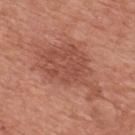The lesion was photographed on a routine skin check and not biopsied; there is no pathology result.
A 15 mm crop from a total-body photograph taken for skin-cancer surveillance.
The lesion-visualizer software estimated a shape eccentricity near 0.8 and two-axis asymmetry of about 0.4. It also reported an average lesion color of about L≈50 a*≈26 b*≈29 (CIELAB). The software also gave a border-irregularity index near 6/10, a color-variation rating of about 3/10, and a peripheral color-asymmetry measure near 1. The software also gave a classifier nevus-likeness of about 35/100 and lesion-presence confidence of about 100/100.
The recorded lesion diameter is about 8 mm.
This is a white-light tile.
A male patient, aged approximately 70.
On the back.